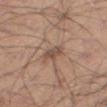Recorded during total-body skin imaging; not selected for excision or biopsy.
A male subject, aged 43–47.
A lesion tile, about 15 mm wide, cut from a 3D total-body photograph.
From the right thigh.
Automated image analysis of the tile measured a mean CIELAB color near L≈50 a*≈18 b*≈26 and a lesion–skin lightness drop of about 9. The analysis additionally found a border-irregularity index near 2.5/10, a within-lesion color-variation index near 2.5/10, and peripheral color asymmetry of about 1. It also reported lesion-presence confidence of about 100/100.
Longest diameter approximately 3 mm.